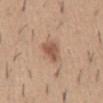Clinical impression: The lesion was photographed on a routine skin check and not biopsied; there is no pathology result. Clinical summary: Captured under white-light illumination. The subject is a male aged around 40. Cropped from a whole-body photographic skin survey; the tile spans about 15 mm. On the abdomen. The lesion's longest dimension is about 3 mm.